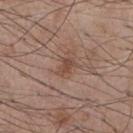  patient:
    sex: male
    age_approx: 55
  automated_metrics:
    shape_asymmetry: 0.35
    nevus_likeness_0_100: 10
    lesion_detection_confidence_0_100: 100
  site: chest
  lighting: white-light
  lesion_size:
    long_diameter_mm_approx: 2.5
  image:
    source: total-body photography crop
    field_of_view_mm: 15